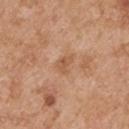Imaged during a routine full-body skin examination; the lesion was not biopsied and no histopathology is available. Automated tile analysis of the lesion measured an area of roughly 3.5 mm² and an eccentricity of roughly 0.7. The software also gave border irregularity of about 3.5 on a 0–10 scale, internal color variation of about 1.5 on a 0–10 scale, and radial color variation of about 0.5. Located on the right upper arm. This is a white-light tile. The patient is a male in their mid- to late 50s. A 15 mm close-up extracted from a 3D total-body photography capture. About 2.5 mm across.